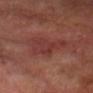Captured during whole-body skin photography for melanoma surveillance; the lesion was not biopsied. The recorded lesion diameter is about 6 mm. The tile uses cross-polarized illumination. Cropped from a total-body skin-imaging series; the visible field is about 15 mm. The patient is a male aged 63 to 67. From the head or neck.